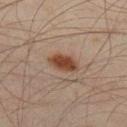Case summary:
- notes: imaged on a skin check; not biopsied
- site: the leg
- illumination: cross-polarized illumination
- patient: male, in their mid- to late 40s
- size: ~4 mm (longest diameter)
- imaging modality: ~15 mm crop, total-body skin-cancer survey